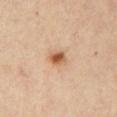The lesion was photographed on a routine skin check and not biopsied; there is no pathology result. From the front of the torso. This is a cross-polarized tile. The lesion-visualizer software estimated a footprint of about 3.5 mm², an outline eccentricity of about 0.5 (0 = round, 1 = elongated), and a symmetry-axis asymmetry near 0.2. The software also gave a normalized border contrast of about 9.5. The software also gave a color-variation rating of about 3.5/10 and peripheral color asymmetry of about 1. The software also gave a detector confidence of about 100 out of 100 that the crop contains a lesion. Measured at roughly 2 mm in maximum diameter. This image is a 15 mm lesion crop taken from a total-body photograph. The patient is a female aged around 40.